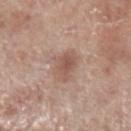{
  "biopsy_status": "not biopsied; imaged during a skin examination",
  "patient": {
    "sex": "male",
    "age_approx": 70
  },
  "lesion_size": {
    "long_diameter_mm_approx": 4.0
  },
  "image": {
    "source": "total-body photography crop",
    "field_of_view_mm": 15
  },
  "lighting": "white-light",
  "site": "leg"
}